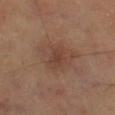Recorded during total-body skin imaging; not selected for excision or biopsy. The lesion is located on the right lower leg. Automated tile analysis of the lesion measured an area of roughly 15 mm², an outline eccentricity of about 0.65 (0 = round, 1 = elongated), and a shape-asymmetry score of about 0.3 (0 = symmetric). Imaged with cross-polarized lighting. A male patient aged around 70. A 15 mm crop from a total-body photograph taken for skin-cancer surveillance.